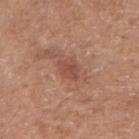  biopsy_status: not biopsied; imaged during a skin examination
  lesion_size:
    long_diameter_mm_approx: 3.0
  lighting: white-light
  image:
    source: total-body photography crop
    field_of_view_mm: 15
  site: right upper arm
  patient:
    sex: male
    age_approx: 65